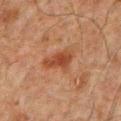Image and clinical context:
The subject is a male in their 60s. Imaged with cross-polarized lighting. About 4 mm across. On the front of the torso. A 15 mm close-up tile from a total-body photography series done for melanoma screening.